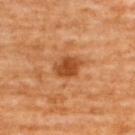{"biopsy_status": "not biopsied; imaged during a skin examination", "site": "upper back", "automated_metrics": {"nevus_likeness_0_100": 80, "lesion_detection_confidence_0_100": 100}, "lesion_size": {"long_diameter_mm_approx": 3.5}, "patient": {"sex": "female", "age_approx": 65}, "lighting": "cross-polarized", "image": {"source": "total-body photography crop", "field_of_view_mm": 15}}